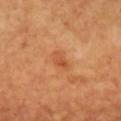workup: no biopsy performed (imaged during a skin exam) | acquisition: ~15 mm tile from a whole-body skin photo | size: about 2.5 mm | patient: female, in their mid- to late 50s | body site: the chest | TBP lesion metrics: a lesion–skin lightness drop of about 8 and a normalized border contrast of about 5.5.